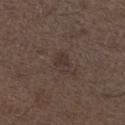Assessment: Imaged during a routine full-body skin examination; the lesion was not biopsied and no histopathology is available. Image and clinical context: The lesion's longest dimension is about 2.5 mm. The subject is a male approximately 50 years of age. Cropped from a whole-body photographic skin survey; the tile spans about 15 mm. Captured under white-light illumination. The lesion is located on the left lower leg.